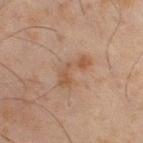Assessment: Part of a total-body skin-imaging series; this lesion was reviewed on a skin check and was not flagged for biopsy. Context: A 15 mm crop from a total-body photograph taken for skin-cancer surveillance. The lesion is located on the right thigh. Imaged with cross-polarized lighting. The patient is a male in their mid- to late 40s. The recorded lesion diameter is about 4.5 mm.